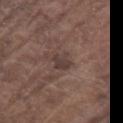Assessment: Captured during whole-body skin photography for melanoma surveillance; the lesion was not biopsied. Clinical summary: The lesion is on the chest. Imaged with white-light lighting. This image is a 15 mm lesion crop taken from a total-body photograph. The patient is a male aged around 80. The lesion-visualizer software estimated an eccentricity of roughly 0.4. It also reported a lesion color around L≈38 a*≈15 b*≈18 in CIELAB and roughly 8 lightness units darker than nearby skin. The software also gave a border-irregularity index near 3/10, a color-variation rating of about 2.5/10, and a peripheral color-asymmetry measure near 1. The analysis additionally found lesion-presence confidence of about 100/100. Approximately 2.5 mm at its widest.